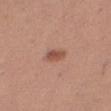location: the right thigh | patient: female, aged 28–32 | imaging modality: ~15 mm tile from a whole-body skin photo.